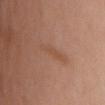The lesion was photographed on a routine skin check and not biopsied; there is no pathology result.
The lesion's longest dimension is about 4 mm.
The lesion is on the mid back.
This is a white-light tile.
An algorithmic analysis of the crop reported a border-irregularity rating of about 5.5/10 and a peripheral color-asymmetry measure near 0. It also reported an automated nevus-likeness rating near 0 out of 100.
A female patient, approximately 65 years of age.
Cropped from a whole-body photographic skin survey; the tile spans about 15 mm.